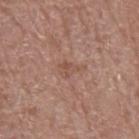Captured during whole-body skin photography for melanoma surveillance; the lesion was not biopsied.
Imaged with white-light lighting.
Located on the left upper arm.
A male subject, in their 70s.
About 3 mm across.
A lesion tile, about 15 mm wide, cut from a 3D total-body photograph.